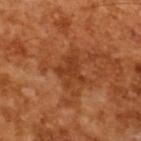Q: Was a biopsy performed?
A: imaged on a skin check; not biopsied
Q: What is the imaging modality?
A: ~15 mm crop, total-body skin-cancer survey
Q: What are the patient's age and sex?
A: male, about 65 years old
Q: Lesion size?
A: ≈4 mm
Q: Automated lesion metrics?
A: a classifier nevus-likeness of about 0/100
Q: What lighting was used for the tile?
A: cross-polarized